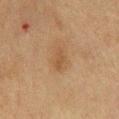Imaged during a routine full-body skin examination; the lesion was not biopsied and no histopathology is available. Longest diameter approximately 3 mm. The total-body-photography lesion software estimated internal color variation of about 1 on a 0–10 scale. The analysis additionally found a detector confidence of about 100 out of 100 that the crop contains a lesion. The lesion is located on the front of the torso. A region of skin cropped from a whole-body photographic capture, roughly 15 mm wide. A male subject aged 73–77. This is a cross-polarized tile.